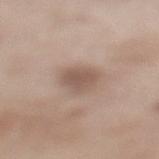Recorded during total-body skin imaging; not selected for excision or biopsy. The lesion's longest dimension is about 3.5 mm. The lesion is located on the left lower leg. The subject is a female aged 53–57. A lesion tile, about 15 mm wide, cut from a 3D total-body photograph.